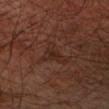Assessment:
This lesion was catalogued during total-body skin photography and was not selected for biopsy.
Background:
This image is a 15 mm lesion crop taken from a total-body photograph. The patient is a male in their mid-60s. The lesion is on the left forearm. The lesion's longest dimension is about 3.5 mm. Captured under cross-polarized illumination.